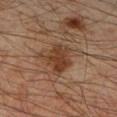Captured during whole-body skin photography for melanoma surveillance; the lesion was not biopsied. Automated tile analysis of the lesion measured a mean CIELAB color near L≈33 a*≈17 b*≈26, about 8 CIELAB-L* units darker than the surrounding skin, and a lesion-to-skin contrast of about 8 (normalized; higher = more distinct). It also reported a classifier nevus-likeness of about 55/100. About 4.5 mm across. The lesion is on the right forearm. A close-up tile cropped from a whole-body skin photograph, about 15 mm across. A male patient aged around 45. Captured under cross-polarized illumination.